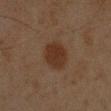Q: Is there a histopathology result?
A: imaged on a skin check; not biopsied
Q: What lighting was used for the tile?
A: cross-polarized
Q: Automated lesion metrics?
A: an average lesion color of about L≈25 a*≈15 b*≈23 (CIELAB) and a normalized lesion–skin contrast near 8.5; border irregularity of about 1 on a 0–10 scale and radial color variation of about 0.5
Q: How was this image acquired?
A: 15 mm crop, total-body photography
Q: What are the patient's age and sex?
A: male, approximately 45 years of age
Q: What is the lesion's diameter?
A: ~3.5 mm (longest diameter)
Q: Lesion location?
A: the left forearm